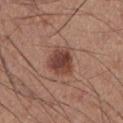biopsy_status: not biopsied; imaged during a skin examination
automated_metrics:
  area_mm2_approx: 9.0
  eccentricity: 0.5
  color_variation_0_10: 5.0
  nevus_likeness_0_100: 90
lighting: white-light
patient:
  sex: male
  age_approx: 35
site: leg
image:
  source: total-body photography crop
  field_of_view_mm: 15
lesion_size:
  long_diameter_mm_approx: 3.5